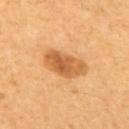notes — no biopsy performed (imaged during a skin exam); image — ~15 mm crop, total-body skin-cancer survey; location — the upper back; subject — male, approximately 60 years of age; diameter — ≈5.5 mm; illumination — cross-polarized; automated metrics — a lesion area of about 12 mm², a shape eccentricity near 0.85, and a shape-asymmetry score of about 0.2 (0 = symmetric).